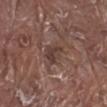Image and clinical context: The total-body-photography lesion software estimated a lesion area of about 5.5 mm² and a symmetry-axis asymmetry near 0.3. And it measured an average lesion color of about L≈39 a*≈17 b*≈21 (CIELAB) and a normalized border contrast of about 7. The software also gave a nevus-likeness score of about 0/100 and lesion-presence confidence of about 95/100. The recorded lesion diameter is about 3 mm. A male patient, aged 78–82. The lesion is located on the leg. A 15 mm close-up tile from a total-body photography series done for melanoma screening. Imaged with white-light lighting.